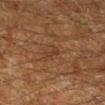{"biopsy_status": "not biopsied; imaged during a skin examination", "site": "right lower leg", "image": {"source": "total-body photography crop", "field_of_view_mm": 15}, "lesion_size": {"long_diameter_mm_approx": 2.5}, "patient": {"sex": "male", "age_approx": 60}}